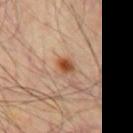The lesion is located on the chest. Cropped from a whole-body photographic skin survey; the tile spans about 15 mm. A male patient approximately 55 years of age.